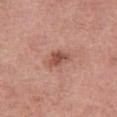{
  "biopsy_status": "not biopsied; imaged during a skin examination",
  "lighting": "white-light",
  "site": "left thigh",
  "patient": {
    "sex": "female",
    "age_approx": 70
  },
  "automated_metrics": {
    "cielab_L": 51,
    "cielab_a": 25,
    "cielab_b": 27,
    "vs_skin_darker_L": 11.0,
    "vs_skin_contrast_norm": 8.0,
    "nevus_likeness_0_100": 50,
    "lesion_detection_confidence_0_100": 100
  },
  "image": {
    "source": "total-body photography crop",
    "field_of_view_mm": 15
  },
  "lesion_size": {
    "long_diameter_mm_approx": 3.0
  }
}